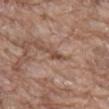  biopsy_status: not biopsied; imaged during a skin examination
  lesion_size:
    long_diameter_mm_approx: 2.5
  image:
    source: total-body photography crop
    field_of_view_mm: 15
  automated_metrics:
    area_mm2_approx: 2.5
    shape_asymmetry: 0.4
    cielab_L: 48
    cielab_a: 19
    cielab_b: 27
    vs_skin_darker_L: 10.0
    nevus_likeness_0_100: 0
    lesion_detection_confidence_0_100: 60
  patient:
    sex: male
    age_approx: 80
  site: abdomen